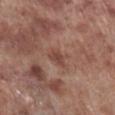Assessment: This lesion was catalogued during total-body skin photography and was not selected for biopsy. Image and clinical context: A 15 mm close-up tile from a total-body photography series done for melanoma screening. The lesion is located on the leg. The tile uses white-light illumination. The subject is a male in their 70s.